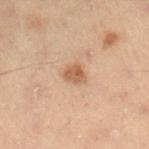{
  "biopsy_status": "not biopsied; imaged during a skin examination",
  "site": "right lower leg",
  "image": {
    "source": "total-body photography crop",
    "field_of_view_mm": 15
  },
  "patient": {
    "sex": "male",
    "age_approx": 55
  }
}